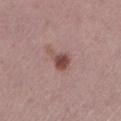Clinical impression: Part of a total-body skin-imaging series; this lesion was reviewed on a skin check and was not flagged for biopsy. Background: A female subject aged around 50. Cropped from a total-body skin-imaging series; the visible field is about 15 mm. The lesion is on the left lower leg.